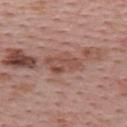workup: catalogued during a skin exam; not biopsied
location: the upper back
automated lesion analysis: a mean CIELAB color near L≈50 a*≈22 b*≈26 and roughly 9 lightness units darker than nearby skin; a border-irregularity index near 3.5/10, a color-variation rating of about 3.5/10, and a peripheral color-asymmetry measure near 1.5; a nevus-likeness score of about 0/100 and lesion-presence confidence of about 65/100
image: ~15 mm crop, total-body skin-cancer survey
subject: female, in their mid- to late 50s
lighting: white-light